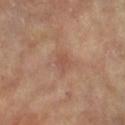Context:
The total-body-photography lesion software estimated internal color variation of about 1 on a 0–10 scale and a peripheral color-asymmetry measure near 0.5. The software also gave a classifier nevus-likeness of about 0/100 and a lesion-detection confidence of about 100/100. A female subject in their mid-70s. Imaged with cross-polarized lighting. Located on the right lower leg. This image is a 15 mm lesion crop taken from a total-body photograph. Longest diameter approximately 3 mm.